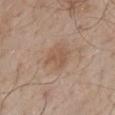Assessment:
Imaged during a routine full-body skin examination; the lesion was not biopsied and no histopathology is available.
Acquisition and patient details:
An algorithmic analysis of the crop reported a border-irregularity index near 3.5/10, internal color variation of about 2 on a 0–10 scale, and peripheral color asymmetry of about 0.5. And it measured an automated nevus-likeness rating near 30 out of 100 and a detector confidence of about 100 out of 100 that the crop contains a lesion. The lesion is on the mid back. A lesion tile, about 15 mm wide, cut from a 3D total-body photograph. Captured under white-light illumination. About 3 mm across. A male patient, about 55 years old.